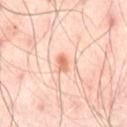<tbp_lesion>
<image>
  <source>total-body photography crop</source>
  <field_of_view_mm>15</field_of_view_mm>
</image>
<site>abdomen</site>
<patient>
  <sex>male</sex>
  <age_approx>50</age_approx>
</patient>
</tbp_lesion>